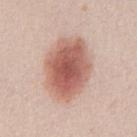<lesion>
  <biopsy_status>not biopsied; imaged during a skin examination</biopsy_status>
  <site>front of the torso</site>
  <image>
    <source>total-body photography crop</source>
    <field_of_view_mm>15</field_of_view_mm>
  </image>
  <lesion_size>
    <long_diameter_mm_approx>7.5</long_diameter_mm_approx>
  </lesion_size>
  <patient>
    <sex>male</sex>
    <age_approx>25</age_approx>
  </patient>
  <automated_metrics>
    <area_mm2_approx>30.0</area_mm2_approx>
    <eccentricity>0.65</eccentricity>
    <lesion_detection_confidence_0_100>100</lesion_detection_confidence_0_100>
  </automated_metrics>
</lesion>